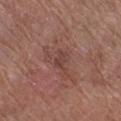No biopsy was performed on this lesion — it was imaged during a full skin examination and was not determined to be concerning. Located on the right lower leg. A 15 mm crop from a total-body photograph taken for skin-cancer surveillance. Captured under white-light illumination. A female subject aged 78 to 82. The total-body-photography lesion software estimated a nevus-likeness score of about 0/100 and a detector confidence of about 100 out of 100 that the crop contains a lesion. Measured at roughly 2.5 mm in maximum diameter.